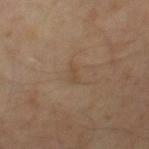Part of a total-body skin-imaging series; this lesion was reviewed on a skin check and was not flagged for biopsy.
This is a cross-polarized tile.
Located on the right thigh.
The patient is a male in their mid-50s.
The lesion-visualizer software estimated an eccentricity of roughly 0.85 and two-axis asymmetry of about 0.35. The software also gave a detector confidence of about 100 out of 100 that the crop contains a lesion.
This image is a 15 mm lesion crop taken from a total-body photograph.